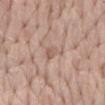Q: Is there a histopathology result?
A: catalogued during a skin exam; not biopsied
Q: What kind of image is this?
A: ~15 mm tile from a whole-body skin photo
Q: What are the patient's age and sex?
A: female, aged 73 to 77
Q: What is the lesion's diameter?
A: about 3.5 mm
Q: Where on the body is the lesion?
A: the mid back
Q: How was the tile lit?
A: white-light illumination